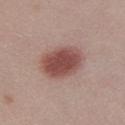Clinical impression:
Recorded during total-body skin imaging; not selected for excision or biopsy.
Clinical summary:
Located on the right lower leg. A female subject aged approximately 20. A roughly 15 mm field-of-view crop from a total-body skin photograph. Longest diameter approximately 5 mm.